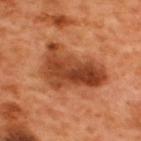Clinical impression:
No biopsy was performed on this lesion — it was imaged during a full skin examination and was not determined to be concerning.